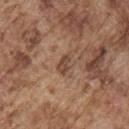Q: Is there a histopathology result?
A: imaged on a skin check; not biopsied
Q: What are the patient's age and sex?
A: male, aged approximately 75
Q: Where on the body is the lesion?
A: the right upper arm
Q: Automated lesion metrics?
A: a border-irregularity rating of about 3/10, a within-lesion color-variation index near 1.5/10, and a peripheral color-asymmetry measure near 0.5
Q: What kind of image is this?
A: ~15 mm crop, total-body skin-cancer survey